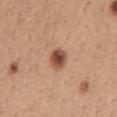Approximately 2.5 mm at its widest. On the chest. This image is a 15 mm lesion crop taken from a total-body photograph. The patient is a female aged 38 to 42.